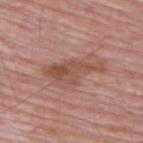Q: Was a biopsy performed?
A: imaged on a skin check; not biopsied
Q: How large is the lesion?
A: about 6.5 mm
Q: What kind of image is this?
A: 15 mm crop, total-body photography
Q: What are the patient's age and sex?
A: male, aged 63 to 67
Q: Illumination type?
A: white-light
Q: Lesion location?
A: the back